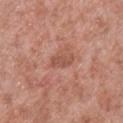Assessment: Imaged during a routine full-body skin examination; the lesion was not biopsied and no histopathology is available. Clinical summary: A male subject, roughly 55 years of age. A roughly 15 mm field-of-view crop from a total-body skin photograph. On the right lower leg.